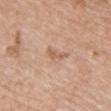{
  "biopsy_status": "not biopsied; imaged during a skin examination",
  "lighting": "white-light",
  "image": {
    "source": "total-body photography crop",
    "field_of_view_mm": 15
  },
  "patient": {
    "sex": "female",
    "age_approx": 40
  },
  "site": "chest",
  "lesion_size": {
    "long_diameter_mm_approx": 3.0
  }
}